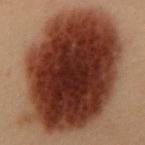Assessment: The lesion was photographed on a routine skin check and not biopsied; there is no pathology result. Acquisition and patient details: A male subject, in their mid- to late 50s. The lesion's longest dimension is about 15 mm. The total-body-photography lesion software estimated an average lesion color of about L≈28 a*≈20 b*≈24 (CIELAB), a lesion–skin lightness drop of about 19, and a normalized border contrast of about 16.5. It also reported internal color variation of about 8 on a 0–10 scale and radial color variation of about 2.5. And it measured a lesion-detection confidence of about 100/100. The lesion is on the front of the torso. Cropped from a whole-body photographic skin survey; the tile spans about 15 mm.